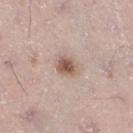Clinical impression:
The lesion was tiled from a total-body skin photograph and was not biopsied.
Clinical summary:
The lesion's longest dimension is about 2.5 mm. From the right thigh. A 15 mm close-up tile from a total-body photography series done for melanoma screening. A male subject, in their mid- to late 50s. The tile uses white-light illumination.